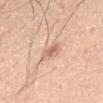No biopsy was performed on this lesion — it was imaged during a full skin examination and was not determined to be concerning. An algorithmic analysis of the crop reported a border-irregularity rating of about 2/10, a within-lesion color-variation index near 4/10, and a peripheral color-asymmetry measure near 1.5. The software also gave an automated nevus-likeness rating near 0 out of 100 and lesion-presence confidence of about 100/100. This is a white-light tile. A male subject aged around 40. Cropped from a total-body skin-imaging series; the visible field is about 15 mm. The lesion is located on the chest.